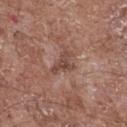Q: Is there a histopathology result?
A: no biopsy performed (imaged during a skin exam)
Q: What is the anatomic site?
A: the chest
Q: How large is the lesion?
A: about 3.5 mm
Q: How was the tile lit?
A: white-light
Q: How was this image acquired?
A: total-body-photography crop, ~15 mm field of view
Q: Who is the patient?
A: male, aged 68–72